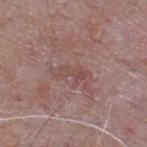Recorded during total-body skin imaging; not selected for excision or biopsy. Imaged with white-light lighting. Automated tile analysis of the lesion measured an area of roughly 3.5 mm², an eccentricity of roughly 0.9, and a symmetry-axis asymmetry near 0.45. The software also gave a lesion–skin lightness drop of about 6 and a lesion-to-skin contrast of about 5 (normalized; higher = more distinct). And it measured an automated nevus-likeness rating near 0 out of 100 and a detector confidence of about 95 out of 100 that the crop contains a lesion. From the back. A lesion tile, about 15 mm wide, cut from a 3D total-body photograph. The recorded lesion diameter is about 3 mm. A male subject aged 63 to 67.